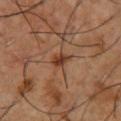Clinical impression: Part of a total-body skin-imaging series; this lesion was reviewed on a skin check and was not flagged for biopsy. Acquisition and patient details: Automated image analysis of the tile measured an eccentricity of roughly 0.75 and two-axis asymmetry of about 0.25. And it measured a mean CIELAB color near L≈38 a*≈22 b*≈30 and roughly 10 lightness units darker than nearby skin. The analysis additionally found border irregularity of about 2 on a 0–10 scale, internal color variation of about 3 on a 0–10 scale, and radial color variation of about 1. A 15 mm close-up extracted from a 3D total-body photography capture. This is a cross-polarized tile. A male subject aged 53–57. The lesion is located on the chest.